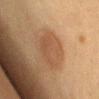On the chest. A lesion tile, about 15 mm wide, cut from a 3D total-body photograph. A female subject approximately 50 years of age.